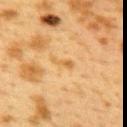No biopsy was performed on this lesion — it was imaged during a full skin examination and was not determined to be concerning. Automated tile analysis of the lesion measured a footprint of about 2.5 mm², a shape eccentricity near 0.95, and a symmetry-axis asymmetry near 0.4. About 3 mm across. A 15 mm crop from a total-body photograph taken for skin-cancer surveillance. Captured under cross-polarized illumination. On the upper back. The subject is a female aged 38 to 42.